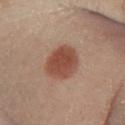Assessment: This lesion was catalogued during total-body skin photography and was not selected for biopsy.